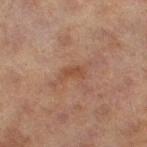location: the leg | subject: female, aged 58 to 62 | size: ≈3 mm | image: total-body-photography crop, ~15 mm field of view.